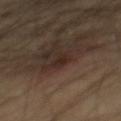notes = catalogued during a skin exam; not biopsied
patient = male, in their mid-50s
acquisition = ~15 mm crop, total-body skin-cancer survey
lesion diameter = about 2.5 mm
illumination = cross-polarized
site = the mid back